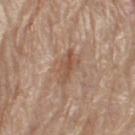The lesion was tiled from a total-body skin photograph and was not biopsied. A male patient approximately 70 years of age. Located on the leg. A 15 mm close-up tile from a total-body photography series done for melanoma screening.